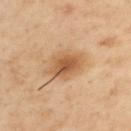Clinical summary:
From the left upper arm. This is a cross-polarized tile. A 15 mm close-up extracted from a 3D total-body photography capture. Longest diameter approximately 5 mm. An algorithmic analysis of the crop reported a footprint of about 11 mm² and an outline eccentricity of about 0.75 (0 = round, 1 = elongated). It also reported a border-irregularity index near 2/10, internal color variation of about 4.5 on a 0–10 scale, and peripheral color asymmetry of about 1.5. It also reported a detector confidence of about 100 out of 100 that the crop contains a lesion. A male patient aged around 55.